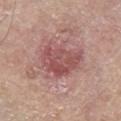{
  "biopsy_status": "not biopsied; imaged during a skin examination",
  "image": {
    "source": "total-body photography crop",
    "field_of_view_mm": 15
  },
  "patient": {
    "sex": "male",
    "age_approx": 70
  },
  "site": "leg",
  "lighting": "white-light"
}